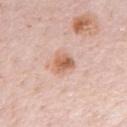The lesion was tiled from a total-body skin photograph and was not biopsied. The patient is a male aged 78–82. The lesion's longest dimension is about 3 mm. Cropped from a whole-body photographic skin survey; the tile spans about 15 mm. From the chest.